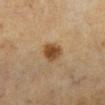automated metrics: an area of roughly 6 mm² and a shape-asymmetry score of about 0.2 (0 = symmetric); a mean CIELAB color near L≈39 a*≈16 b*≈31, about 11 CIELAB-L* units darker than the surrounding skin, and a lesion-to-skin contrast of about 9.5 (normalized; higher = more distinct); a border-irregularity rating of about 1.5/10, a color-variation rating of about 3.5/10, and peripheral color asymmetry of about 1; a classifier nevus-likeness of about 95/100 and a detector confidence of about 100 out of 100 that the crop contains a lesion
image source: ~15 mm tile from a whole-body skin photo
location: the left lower leg
subject: female, aged around 55